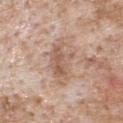A male patient, approximately 65 years of age. A lesion tile, about 15 mm wide, cut from a 3D total-body photograph. This is a white-light tile. The lesion is on the chest. An algorithmic analysis of the crop reported a mean CIELAB color near L≈59 a*≈18 b*≈28 and a normalized lesion–skin contrast near 6.5. It also reported a detector confidence of about 85 out of 100 that the crop contains a lesion.